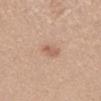Automated image analysis of the tile measured roughly 9 lightness units darker than nearby skin and a lesion-to-skin contrast of about 6 (normalized; higher = more distinct). The software also gave internal color variation of about 0.5 on a 0–10 scale and a peripheral color-asymmetry measure near 0. And it measured a classifier nevus-likeness of about 40/100 and lesion-presence confidence of about 100/100. Located on the mid back. Measured at roughly 2.5 mm in maximum diameter. Cropped from a whole-body photographic skin survey; the tile spans about 15 mm. The tile uses white-light illumination. The patient is a female roughly 40 years of age.